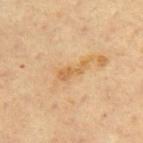Impression:
Captured during whole-body skin photography for melanoma surveillance; the lesion was not biopsied.
Image and clinical context:
A 15 mm close-up extracted from a 3D total-body photography capture. Captured under cross-polarized illumination. The total-body-photography lesion software estimated an area of roughly 3.5 mm² and a shape eccentricity near 0.95. And it measured about 7 CIELAB-L* units darker than the surrounding skin and a normalized lesion–skin contrast near 6.5. It also reported a classifier nevus-likeness of about 0/100 and a detector confidence of about 100 out of 100 that the crop contains a lesion. From the mid back. Measured at roughly 3.5 mm in maximum diameter. A male subject roughly 65 years of age.